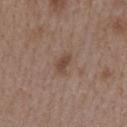Notes:
– workup · no biopsy performed (imaged during a skin exam)
– image source · ~15 mm tile from a whole-body skin photo
– patient · female, in their mid- to late 40s
– location · the upper back